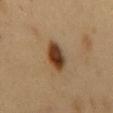This lesion was catalogued during total-body skin photography and was not selected for biopsy. The total-body-photography lesion software estimated a lesion area of about 8 mm², a shape eccentricity near 0.8, and a symmetry-axis asymmetry near 0.25. The software also gave an automated nevus-likeness rating near 100 out of 100 and lesion-presence confidence of about 100/100. About 4 mm across. A male patient, aged 48 to 52. A roughly 15 mm field-of-view crop from a total-body skin photograph. Imaged with cross-polarized lighting. The lesion is on the front of the torso.